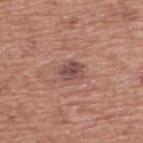Q: Was this lesion biopsied?
A: no biopsy performed (imaged during a skin exam)
Q: Where on the body is the lesion?
A: the upper back
Q: What is the lesion's diameter?
A: ≈2.5 mm
Q: What lighting was used for the tile?
A: white-light
Q: Automated lesion metrics?
A: an average lesion color of about L≈48 a*≈22 b*≈22 (CIELAB), about 10 CIELAB-L* units darker than the surrounding skin, and a normalized border contrast of about 8; peripheral color asymmetry of about 1.5
Q: Patient demographics?
A: male, aged 73 to 77
Q: What is the imaging modality?
A: ~15 mm crop, total-body skin-cancer survey